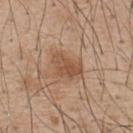No biopsy was performed on this lesion — it was imaged during a full skin examination and was not determined to be concerning. This is a white-light tile. A male patient, in their mid- to late 50s. A 15 mm close-up tile from a total-body photography series done for melanoma screening. The lesion's longest dimension is about 4 mm. On the upper back.